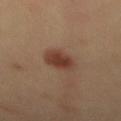The lesion was photographed on a routine skin check and not biopsied; there is no pathology result.
A close-up tile cropped from a whole-body skin photograph, about 15 mm across.
The lesion is on the mid back.
A male patient, aged around 50.
The total-body-photography lesion software estimated a mean CIELAB color near L≈38 a*≈20 b*≈26, roughly 10 lightness units darker than nearby skin, and a lesion-to-skin contrast of about 9 (normalized; higher = more distinct). The analysis additionally found a border-irregularity rating of about 2/10 and a color-variation rating of about 3.5/10. It also reported lesion-presence confidence of about 100/100.
The lesion's longest dimension is about 3.5 mm.